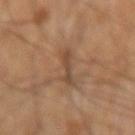Q: Was a biopsy performed?
A: no biopsy performed (imaged during a skin exam)
Q: What kind of image is this?
A: 15 mm crop, total-body photography
Q: Illumination type?
A: cross-polarized illumination
Q: What is the anatomic site?
A: the right upper arm
Q: Automated lesion metrics?
A: an area of roughly 4 mm², a shape eccentricity near 0.9, and two-axis asymmetry of about 0.25; an average lesion color of about L≈46 a*≈17 b*≈30 (CIELAB) and about 8 CIELAB-L* units darker than the surrounding skin; a nevus-likeness score of about 0/100
Q: What is the lesion's diameter?
A: ≈3 mm
Q: What are the patient's age and sex?
A: male, aged 53 to 57